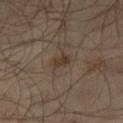The lesion was tiled from a total-body skin photograph and was not biopsied. Captured under cross-polarized illumination. The lesion is on the right thigh. The lesion-visualizer software estimated a lesion area of about 3.5 mm² and an eccentricity of roughly 0.8. It also reported a mean CIELAB color near L≈33 a*≈12 b*≈24 and about 6 CIELAB-L* units darker than the surrounding skin. And it measured a border-irregularity rating of about 2/10, a color-variation rating of about 2.5/10, and peripheral color asymmetry of about 1. The software also gave an automated nevus-likeness rating near 0 out of 100 and a detector confidence of about 100 out of 100 that the crop contains a lesion. Cropped from a whole-body photographic skin survey; the tile spans about 15 mm. A male subject aged approximately 65.